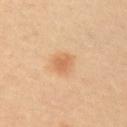Clinical impression: Captured during whole-body skin photography for melanoma surveillance; the lesion was not biopsied. Background: Imaged with cross-polarized lighting. The lesion-visualizer software estimated border irregularity of about 3 on a 0–10 scale, internal color variation of about 2 on a 0–10 scale, and radial color variation of about 0.5. The analysis additionally found a classifier nevus-likeness of about 80/100 and a detector confidence of about 100 out of 100 that the crop contains a lesion. A male patient, in their 40s. A 15 mm close-up extracted from a 3D total-body photography capture. On the right upper arm. Approximately 3 mm at its widest.